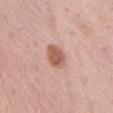biopsy status — catalogued during a skin exam; not biopsied
image — total-body-photography crop, ~15 mm field of view
location — the abdomen
patient — female, aged 63 to 67
size — ≈3.5 mm
lighting — white-light